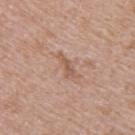Findings:
* workup: catalogued during a skin exam; not biopsied
* location: the upper back
* tile lighting: white-light
* lesion diameter: about 3.5 mm
* image source: 15 mm crop, total-body photography
* subject: female, about 40 years old